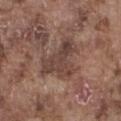Context:
The lesion is on the right thigh. Imaged with white-light lighting. A 15 mm crop from a total-body photograph taken for skin-cancer surveillance. A male patient aged 73 to 77.